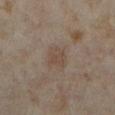• biopsy status — catalogued during a skin exam; not biopsied
• imaging modality — 15 mm crop, total-body photography
• diameter — about 3 mm
• patient — female, in their mid- to late 30s
• image-analysis metrics — an outline eccentricity of about 0.65 (0 = round, 1 = elongated) and a symmetry-axis asymmetry near 0.3; an average lesion color of about L≈43 a*≈13 b*≈22 (CIELAB), about 6 CIELAB-L* units darker than the surrounding skin, and a normalized lesion–skin contrast near 5; a border-irregularity rating of about 2.5/10, a within-lesion color-variation index near 2/10, and peripheral color asymmetry of about 0.5; a nevus-likeness score of about 20/100
• body site — the left lower leg
• illumination — cross-polarized illumination